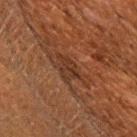Clinical impression: The lesion was photographed on a routine skin check and not biopsied; there is no pathology result. Acquisition and patient details: The subject is a female aged approximately 50. The lesion is located on the back. A 15 mm close-up tile from a total-body photography series done for melanoma screening.